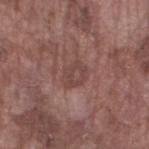Image and clinical context: The subject is a male aged approximately 75. A roughly 15 mm field-of-view crop from a total-body skin photograph. Approximately 3 mm at its widest. Imaged with white-light lighting. Located on the left thigh.